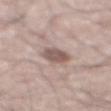Assessment:
The lesion was photographed on a routine skin check and not biopsied; there is no pathology result.
Context:
A male patient roughly 65 years of age. Located on the abdomen. A roughly 15 mm field-of-view crop from a total-body skin photograph. The lesion-visualizer software estimated a mean CIELAB color near L≈55 a*≈16 b*≈20. Approximately 3.5 mm at its widest.